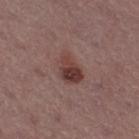biopsy status = imaged on a skin check; not biopsied | acquisition = ~15 mm crop, total-body skin-cancer survey | subject = female, approximately 55 years of age | site = the right thigh.